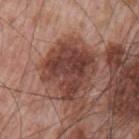Imaged during a routine full-body skin examination; the lesion was not biopsied and no histopathology is available.
The lesion is on the arm.
A male patient aged around 65.
Longest diameter approximately 6 mm.
The total-body-photography lesion software estimated a color-variation rating of about 4.5/10 and peripheral color asymmetry of about 1.5. The software also gave an automated nevus-likeness rating near 5 out of 100 and a detector confidence of about 100 out of 100 that the crop contains a lesion.
Cropped from a whole-body photographic skin survey; the tile spans about 15 mm.